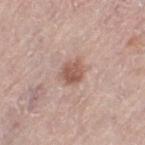workup — total-body-photography surveillance lesion; no biopsy
subject — female, about 65 years old
image — total-body-photography crop, ~15 mm field of view
location — the left thigh